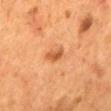This lesion was catalogued during total-body skin photography and was not selected for biopsy. The patient is a male aged around 55. A close-up tile cropped from a whole-body skin photograph, about 15 mm across. The lesion is on the mid back. Approximately 3 mm at its widest.